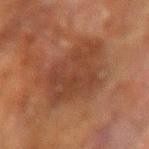<record>
  <biopsy_status>not biopsied; imaged during a skin examination</biopsy_status>
  <lighting>cross-polarized</lighting>
  <patient>
    <sex>male</sex>
    <age_approx>60</age_approx>
  </patient>
  <site>left arm</site>
  <image>
    <source>total-body photography crop</source>
    <field_of_view_mm>15</field_of_view_mm>
  </image>
</record>